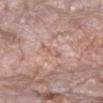Clinical impression: Imaged during a routine full-body skin examination; the lesion was not biopsied and no histopathology is available. Context: A 15 mm close-up tile from a total-body photography series done for melanoma screening. An algorithmic analysis of the crop reported lesion-presence confidence of about 100/100. Captured under white-light illumination. On the right forearm. A female patient, aged 68 to 72.